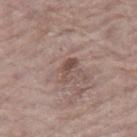{"biopsy_status": "not biopsied; imaged during a skin examination", "automated_metrics": {"border_irregularity_0_10": 5.5, "color_variation_0_10": 1.0, "nevus_likeness_0_100": 0, "lesion_detection_confidence_0_100": 100}, "image": {"source": "total-body photography crop", "field_of_view_mm": 15}, "site": "leg", "lighting": "white-light", "patient": {"sex": "female", "age_approx": "85+"}}